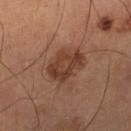biopsy status: catalogued during a skin exam; not biopsied | body site: the right lower leg | patient: male, approximately 60 years of age | image: 15 mm crop, total-body photography.